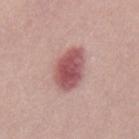Impression: Part of a total-body skin-imaging series; this lesion was reviewed on a skin check and was not flagged for biopsy. Acquisition and patient details: Measured at roughly 5 mm in maximum diameter. A male subject, aged around 30. The tile uses white-light illumination. A region of skin cropped from a whole-body photographic capture, roughly 15 mm wide.